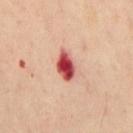workup=catalogued during a skin exam; not biopsied
patient=male, approximately 55 years of age
image=15 mm crop, total-body photography
body site=the back
image-analysis metrics=a footprint of about 6.5 mm², a shape eccentricity near 0.8, and two-axis asymmetry of about 0.15; an average lesion color of about L≈52 a*≈38 b*≈28 (CIELAB); a classifier nevus-likeness of about 0/100 and lesion-presence confidence of about 100/100
illumination=cross-polarized illumination
lesion diameter=about 3.5 mm